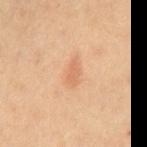<record>
<biopsy_status>not biopsied; imaged during a skin examination</biopsy_status>
<patient>
  <sex>male</sex>
  <age_approx>70</age_approx>
</patient>
<automated_metrics>
  <eccentricity>0.9</eccentricity>
  <cielab_L>63</cielab_L>
  <cielab_a>22</cielab_a>
  <cielab_b>35</cielab_b>
  <vs_skin_darker_L>7.0</vs_skin_darker_L>
  <vs_skin_contrast_norm>5.0</vs_skin_contrast_norm>
  <nevus_likeness_0_100>15</nevus_likeness_0_100>
  <lesion_detection_confidence_0_100>100</lesion_detection_confidence_0_100>
</automated_metrics>
<image>
  <source>total-body photography crop</source>
  <field_of_view_mm>15</field_of_view_mm>
</image>
<site>abdomen</site>
<lighting>cross-polarized</lighting>
<lesion_size>
  <long_diameter_mm_approx>3.5</long_diameter_mm_approx>
</lesion_size>
</record>